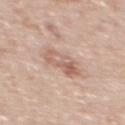Findings:
– workup · total-body-photography surveillance lesion; no biopsy
– patient · male, aged around 60
– lesion diameter · about 4 mm
– location · the upper back
– TBP lesion metrics · a border-irregularity index near 2.5/10 and a color-variation rating of about 7.5/10
– acquisition · total-body-photography crop, ~15 mm field of view
– lighting · white-light illumination